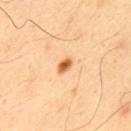This lesion was catalogued during total-body skin photography and was not selected for biopsy. This is a cross-polarized tile. The subject is a male aged 63 to 67. Located on the upper back. An algorithmic analysis of the crop reported a nevus-likeness score of about 100/100 and lesion-presence confidence of about 100/100. Cropped from a whole-body photographic skin survey; the tile spans about 15 mm.